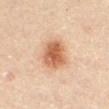Impression: The lesion was tiled from a total-body skin photograph and was not biopsied. Acquisition and patient details: Cropped from a whole-body photographic skin survey; the tile spans about 15 mm. The patient is a male aged around 85 or older. The lesion is on the abdomen.